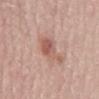Impression:
Captured during whole-body skin photography for melanoma surveillance; the lesion was not biopsied.
Context:
The tile uses white-light illumination. A female patient, aged approximately 65. A 15 mm close-up extracted from a 3D total-body photography capture. Approximately 5.5 mm at its widest. The lesion is located on the mid back.